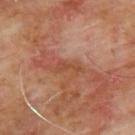Notes:
- workup — catalogued during a skin exam; not biopsied
- location — the back
- subject — male, aged 63 to 67
- imaging modality — 15 mm crop, total-body photography
- image-analysis metrics — an area of roughly 3.5 mm² and a shape eccentricity near 0.9; a mean CIELAB color near L≈46 a*≈23 b*≈32, a lesion–skin lightness drop of about 7, and a normalized border contrast of about 6; a classifier nevus-likeness of about 0/100
- size — ~3.5 mm (longest diameter)
- illumination — cross-polarized illumination